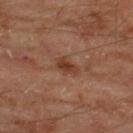Findings:
• body site — the back
• illumination — cross-polarized illumination
• acquisition — 15 mm crop, total-body photography
• lesion diameter — about 3 mm
• patient — male, aged 63 to 67